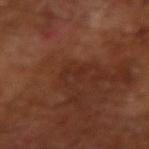• workup — catalogued during a skin exam; not biopsied
• subject — male, about 65 years old
• image source — ~15 mm tile from a whole-body skin photo
• lighting — cross-polarized illumination
• lesion diameter — about 3 mm